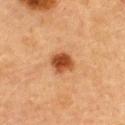{
  "biopsy_status": "not biopsied; imaged during a skin examination",
  "site": "upper back",
  "image": {
    "source": "total-body photography crop",
    "field_of_view_mm": 15
  },
  "patient": {
    "sex": "female",
    "age_approx": 60
  },
  "lighting": "cross-polarized",
  "lesion_size": {
    "long_diameter_mm_approx": 2.5
  }
}